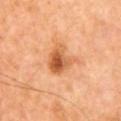Notes:
• biopsy status — no biopsy performed (imaged during a skin exam)
• lesion size — ≈4 mm
• patient — male, aged 63–67
• image source — 15 mm crop, total-body photography
• site — the chest
• tile lighting — cross-polarized
• TBP lesion metrics — a shape eccentricity near 0.45 and a symmetry-axis asymmetry near 0.3; a mean CIELAB color near L≈57 a*≈26 b*≈40 and a normalized lesion–skin contrast near 8; a classifier nevus-likeness of about 65/100 and a lesion-detection confidence of about 100/100